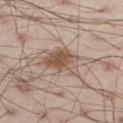Recorded during total-body skin imaging; not selected for excision or biopsy. The patient is a male in their 30s. Located on the left thigh. A roughly 15 mm field-of-view crop from a total-body skin photograph.